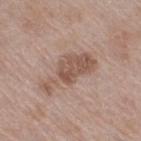Findings:
* follow-up: total-body-photography surveillance lesion; no biopsy
* lesion size: ≈7 mm
* patient: female, aged 38 to 42
* TBP lesion metrics: an outline eccentricity of about 0.9 (0 = round, 1 = elongated); a mean CIELAB color near L≈53 a*≈18 b*≈26 and a normalized border contrast of about 7.5; internal color variation of about 3 on a 0–10 scale and radial color variation of about 1; an automated nevus-likeness rating near 5 out of 100 and lesion-presence confidence of about 100/100
* tile lighting: white-light illumination
* site: the right thigh
* imaging modality: ~15 mm tile from a whole-body skin photo